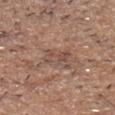Q: What did automated image analysis measure?
A: an eccentricity of roughly 0.85; an automated nevus-likeness rating near 0 out of 100 and lesion-presence confidence of about 85/100
Q: What kind of image is this?
A: total-body-photography crop, ~15 mm field of view
Q: What is the lesion's diameter?
A: about 3.5 mm
Q: What is the anatomic site?
A: the head or neck
Q: Patient demographics?
A: male, aged 68–72